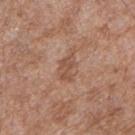workup: total-body-photography surveillance lesion; no biopsy | imaging modality: total-body-photography crop, ~15 mm field of view | patient: male, aged approximately 55 | site: the right forearm.